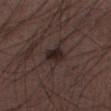Case summary:
* biopsy status — total-body-photography surveillance lesion; no biopsy
* anatomic site — the left lower leg
* subject — male, aged 48–52
* tile lighting — white-light
* size — ~2.5 mm (longest diameter)
* imaging modality — 15 mm crop, total-body photography